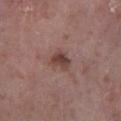{
  "biopsy_status": "not biopsied; imaged during a skin examination",
  "lighting": "white-light",
  "automated_metrics": {
    "area_mm2_approx": 5.0,
    "eccentricity": 0.6,
    "shape_asymmetry": 0.3,
    "nevus_likeness_0_100": 55,
    "lesion_detection_confidence_0_100": 100
  },
  "lesion_size": {
    "long_diameter_mm_approx": 2.5
  },
  "patient": {
    "sex": "male",
    "age_approx": 55
  },
  "site": "right lower leg",
  "image": {
    "source": "total-body photography crop",
    "field_of_view_mm": 15
  }
}